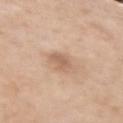A 15 mm crop from a total-body photograph taken for skin-cancer surveillance.
The tile uses white-light illumination.
The lesion is located on the left upper arm.
A female patient, aged approximately 55.
About 2.5 mm across.